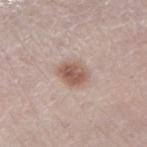| key | value |
|---|---|
| follow-up | catalogued during a skin exam; not biopsied |
| tile lighting | white-light |
| imaging modality | ~15 mm crop, total-body skin-cancer survey |
| subject | male, aged approximately 65 |
| automated metrics | an average lesion color of about L≈56 a*≈18 b*≈25 (CIELAB) and roughly 12 lightness units darker than nearby skin; a nevus-likeness score of about 90/100 and a detector confidence of about 100 out of 100 that the crop contains a lesion |
| lesion size | about 3.5 mm |
| site | the right lower leg |